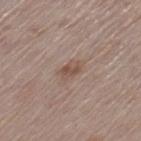biopsy status: catalogued during a skin exam; not biopsied | automated metrics: an area of roughly 3.5 mm², a shape eccentricity near 0.8, and a symmetry-axis asymmetry near 0.3; an average lesion color of about L≈50 a*≈17 b*≈25 (CIELAB), a lesion–skin lightness drop of about 8, and a lesion-to-skin contrast of about 6.5 (normalized; higher = more distinct); an automated nevus-likeness rating near 25 out of 100 and lesion-presence confidence of about 100/100 | diameter: ~2.5 mm (longest diameter) | site: the left thigh | patient: female, about 65 years old | acquisition: ~15 mm tile from a whole-body skin photo.